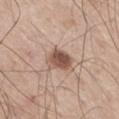The lesion is on the right thigh. A 15 mm crop from a total-body photograph taken for skin-cancer surveillance. Automated tile analysis of the lesion measured an outline eccentricity of about 0.6 (0 = round, 1 = elongated) and a symmetry-axis asymmetry near 0.2. The software also gave a border-irregularity rating of about 2/10 and a within-lesion color-variation index near 5/10. Captured under white-light illumination. Longest diameter approximately 3 mm. A male subject in their 60s.